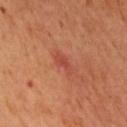Impression: Captured during whole-body skin photography for melanoma surveillance; the lesion was not biopsied. Context: On the upper back. A 15 mm crop from a total-body photograph taken for skin-cancer surveillance. A male patient aged 68 to 72. Imaged with cross-polarized lighting. The lesion-visualizer software estimated a lesion-to-skin contrast of about 5.5 (normalized; higher = more distinct). The software also gave a within-lesion color-variation index near 1/10 and a peripheral color-asymmetry measure near 0.